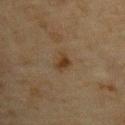Q: How was the tile lit?
A: cross-polarized
Q: Lesion location?
A: the chest
Q: What is the imaging modality?
A: total-body-photography crop, ~15 mm field of view
Q: Patient demographics?
A: male, aged around 65